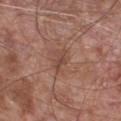Part of a total-body skin-imaging series; this lesion was reviewed on a skin check and was not flagged for biopsy. Longest diameter approximately 3 mm. The subject is a male aged 63 to 67. On the chest. A close-up tile cropped from a whole-body skin photograph, about 15 mm across. Automated tile analysis of the lesion measured a classifier nevus-likeness of about 0/100 and a detector confidence of about 100 out of 100 that the crop contains a lesion. Imaged with white-light lighting.